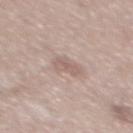The lesion was tiled from a total-body skin photograph and was not biopsied. Measured at roughly 2.5 mm in maximum diameter. A male patient, aged 53–57. Cropped from a total-body skin-imaging series; the visible field is about 15 mm. An algorithmic analysis of the crop reported an area of roughly 3.5 mm², an outline eccentricity of about 0.8 (0 = round, 1 = elongated), and a symmetry-axis asymmetry near 0.4. The analysis additionally found an automated nevus-likeness rating near 0 out of 100.